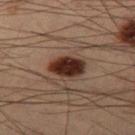No biopsy was performed on this lesion — it was imaged during a full skin examination and was not determined to be concerning. Located on the left thigh. A region of skin cropped from a whole-body photographic capture, roughly 15 mm wide. This is a cross-polarized tile. Approximately 3.5 mm at its widest. A male subject approximately 55 years of age.